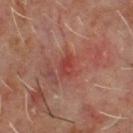Assessment:
Part of a total-body skin-imaging series; this lesion was reviewed on a skin check and was not flagged for biopsy.
Context:
This is a cross-polarized tile. Cropped from a whole-body photographic skin survey; the tile spans about 15 mm. A male patient, aged 58 to 62. Automated image analysis of the tile measured border irregularity of about 3 on a 0–10 scale. The analysis additionally found an automated nevus-likeness rating near 0 out of 100 and a detector confidence of about 100 out of 100 that the crop contains a lesion. Measured at roughly 2.5 mm in maximum diameter. The lesion is located on the chest.